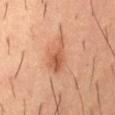Impression: Imaged during a routine full-body skin examination; the lesion was not biopsied and no histopathology is available. Background: The subject is a male approximately 55 years of age. This image is a 15 mm lesion crop taken from a total-body photograph. From the lower back.